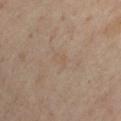Findings:
– follow-up: no biopsy performed (imaged during a skin exam)
– size: ≈2 mm
– imaging modality: total-body-photography crop, ~15 mm field of view
– location: the right upper arm
– automated metrics: a border-irregularity index near 3.5/10 and a color-variation rating of about 1/10
– subject: male, approximately 45 years of age